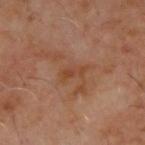No biopsy was performed on this lesion — it was imaged during a full skin examination and was not determined to be concerning. A male patient aged 58 to 62. The lesion's longest dimension is about 3 mm. Imaged with cross-polarized lighting. From the upper back. A region of skin cropped from a whole-body photographic capture, roughly 15 mm wide.